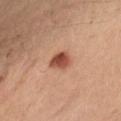Findings:
– workup: imaged on a skin check; not biopsied
– anatomic site: the left lower leg
– imaging modality: total-body-photography crop, ~15 mm field of view
– patient: female, in their mid- to late 30s
– lesion size: about 3 mm